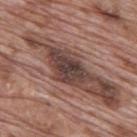The lesion was photographed on a routine skin check and not biopsied; there is no pathology result. The lesion-visualizer software estimated a footprint of about 11 mm² and a shape-asymmetry score of about 0.35 (0 = symmetric). The analysis additionally found internal color variation of about 4 on a 0–10 scale. And it measured lesion-presence confidence of about 60/100. Captured under white-light illumination. From the mid back. The lesion's longest dimension is about 6 mm. A lesion tile, about 15 mm wide, cut from a 3D total-body photograph. A male patient, approximately 70 years of age.